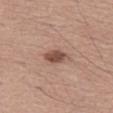No biopsy was performed on this lesion — it was imaged during a full skin examination and was not determined to be concerning.
The lesion's longest dimension is about 3.5 mm.
A roughly 15 mm field-of-view crop from a total-body skin photograph.
This is a white-light tile.
The lesion is located on the abdomen.
The subject is a male aged 53–57.